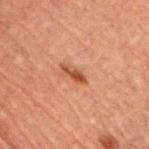{
  "biopsy_status": "not biopsied; imaged during a skin examination",
  "patient": {
    "sex": "male",
    "age_approx": 60
  },
  "image": {
    "source": "total-body photography crop",
    "field_of_view_mm": 15
  },
  "lighting": "cross-polarized",
  "site": "chest",
  "lesion_size": {
    "long_diameter_mm_approx": 3.0
  }
}